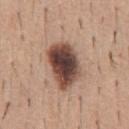Context:
On the chest. Cropped from a total-body skin-imaging series; the visible field is about 15 mm. Approximately 6 mm at its widest. Captured under white-light illumination. The patient is a male approximately 40 years of age.